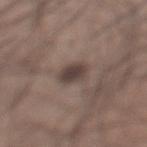workup=imaged on a skin check; not biopsied | lesion diameter=≈3.5 mm | lighting=white-light | imaging modality=15 mm crop, total-body photography | automated metrics=a classifier nevus-likeness of about 75/100 and a lesion-detection confidence of about 100/100 | subject=male, aged 63–67 | anatomic site=the left lower leg.